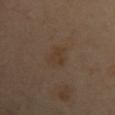The lesion was photographed on a routine skin check and not biopsied; there is no pathology result. A lesion tile, about 15 mm wide, cut from a 3D total-body photograph. Longest diameter approximately 4 mm. Located on the left upper arm. The subject is a female in their 40s. Captured under cross-polarized illumination.